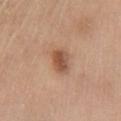biopsy status — catalogued during a skin exam; not biopsied | subject — female, aged approximately 55 | image source — ~15 mm tile from a whole-body skin photo | lesion size — ~3 mm (longest diameter) | automated metrics — an area of roughly 5.5 mm² and a shape-asymmetry score of about 0.2 (0 = symmetric); a mean CIELAB color near L≈52 a*≈22 b*≈31, a lesion–skin lightness drop of about 12, and a normalized border contrast of about 8.5 | site — the chest.